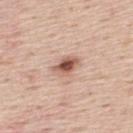Assessment: Imaged during a routine full-body skin examination; the lesion was not biopsied and no histopathology is available. Context: The patient is a male approximately 45 years of age. The lesion's longest dimension is about 3.5 mm. A region of skin cropped from a whole-body photographic capture, roughly 15 mm wide. The lesion is on the mid back. Captured under white-light illumination. The lesion-visualizer software estimated an average lesion color of about L≈57 a*≈22 b*≈28 (CIELAB), about 15 CIELAB-L* units darker than the surrounding skin, and a normalized border contrast of about 9.5. It also reported an automated nevus-likeness rating near 95 out of 100 and a detector confidence of about 100 out of 100 that the crop contains a lesion.